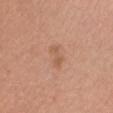Clinical impression: The lesion was photographed on a routine skin check and not biopsied; there is no pathology result. Acquisition and patient details: From the head or neck. Cropped from a whole-body photographic skin survey; the tile spans about 15 mm. Captured under white-light illumination. Measured at roughly 3 mm in maximum diameter. The subject is a female about 30 years old.